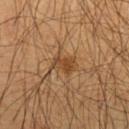{
  "biopsy_status": "not biopsied; imaged during a skin examination",
  "lesion_size": {
    "long_diameter_mm_approx": 3.0
  },
  "image": {
    "source": "total-body photography crop",
    "field_of_view_mm": 15
  },
  "patient": {
    "sex": "male",
    "age_approx": 60
  },
  "site": "front of the torso"
}